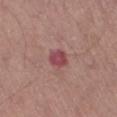Captured during whole-body skin photography for melanoma surveillance; the lesion was not biopsied. On the left thigh. The subject is a male approximately 70 years of age. Cropped from a total-body skin-imaging series; the visible field is about 15 mm.